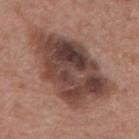| key | value |
|---|---|
| workup | no biopsy performed (imaged during a skin exam) |
| body site | the mid back |
| subject | female, aged around 75 |
| acquisition | 15 mm crop, total-body photography |